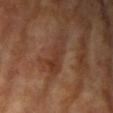Q: Is there a histopathology result?
A: no biopsy performed (imaged during a skin exam)
Q: How was this image acquired?
A: ~15 mm tile from a whole-body skin photo
Q: Who is the patient?
A: female, approximately 75 years of age
Q: Where on the body is the lesion?
A: the left upper arm